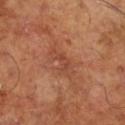biopsy status=total-body-photography surveillance lesion; no biopsy
anatomic site=the right lower leg
tile lighting=cross-polarized
patient=male, approximately 65 years of age
image source=~15 mm crop, total-body skin-cancer survey
size=~4 mm (longest diameter)